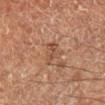| key | value |
|---|---|
| notes | total-body-photography surveillance lesion; no biopsy |
| automated metrics | a footprint of about 4.5 mm², an outline eccentricity of about 0.65 (0 = round, 1 = elongated), and a shape-asymmetry score of about 0.35 (0 = symmetric); an automated nevus-likeness rating near 0 out of 100 and a detector confidence of about 100 out of 100 that the crop contains a lesion |
| imaging modality | ~15 mm crop, total-body skin-cancer survey |
| subject | male, aged around 60 |
| lesion diameter | ≈3 mm |
| site | the right leg |